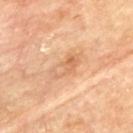Q: Was a biopsy performed?
A: imaged on a skin check; not biopsied